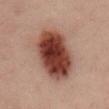Q: Is there a histopathology result?
A: no biopsy performed (imaged during a skin exam)
Q: Lesion location?
A: the back
Q: Illumination type?
A: cross-polarized illumination
Q: Patient demographics?
A: male, aged 48 to 52
Q: What is the lesion's diameter?
A: about 8.5 mm
Q: What did automated image analysis measure?
A: a within-lesion color-variation index near 8.5/10 and radial color variation of about 3
Q: How was this image acquired?
A: ~15 mm crop, total-body skin-cancer survey